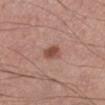Clinical impression:
Recorded during total-body skin imaging; not selected for excision or biopsy.
Acquisition and patient details:
The lesion-visualizer software estimated a lesion area of about 4 mm² and a shape eccentricity near 0.75. And it measured a lesion–skin lightness drop of about 11. And it measured border irregularity of about 2 on a 0–10 scale and a color-variation rating of about 2.5/10. Cropped from a total-body skin-imaging series; the visible field is about 15 mm. Located on the left lower leg. This is a white-light tile. A male patient, aged approximately 40. Measured at roughly 2.5 mm in maximum diameter.